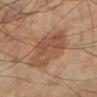biopsy status: catalogued during a skin exam; not biopsied
lesion diameter: about 5.5 mm
acquisition: 15 mm crop, total-body photography
illumination: cross-polarized illumination
subject: male, about 60 years old
site: the right lower leg